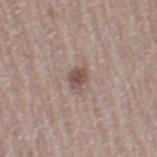The lesion was tiled from a total-body skin photograph and was not biopsied.
A close-up tile cropped from a whole-body skin photograph, about 15 mm across.
A female subject aged around 65.
About 2.5 mm across.
On the leg.
Imaged with white-light lighting.